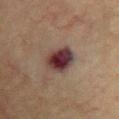No biopsy was performed on this lesion — it was imaged during a full skin examination and was not determined to be concerning.
Captured under cross-polarized illumination.
Approximately 4 mm at its widest.
On the chest.
A region of skin cropped from a whole-body photographic capture, roughly 15 mm wide.
A male subject, aged 73–77.